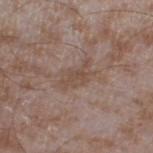Impression: The lesion was photographed on a routine skin check and not biopsied; there is no pathology result. Context: The lesion is located on the right thigh. The lesion's longest dimension is about 4.5 mm. A lesion tile, about 15 mm wide, cut from a 3D total-body photograph. The patient is a male in their mid- to late 40s. Captured under white-light illumination. Automated image analysis of the tile measured about 6 CIELAB-L* units darker than the surrounding skin.